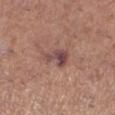Captured during whole-body skin photography for melanoma surveillance; the lesion was not biopsied.
The lesion is on the left lower leg.
A roughly 15 mm field-of-view crop from a total-body skin photograph.
Approximately 3.5 mm at its widest.
The subject is a female approximately 65 years of age.